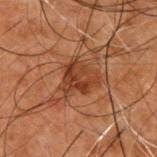The lesion was photographed on a routine skin check and not biopsied; there is no pathology result.
On the upper back.
A male subject, aged 48 to 52.
A roughly 15 mm field-of-view crop from a total-body skin photograph.
The tile uses cross-polarized illumination.
The lesion's longest dimension is about 4 mm.
The lesion-visualizer software estimated an average lesion color of about L≈31 a*≈21 b*≈28 (CIELAB), about 7 CIELAB-L* units darker than the surrounding skin, and a lesion-to-skin contrast of about 7.5 (normalized; higher = more distinct). And it measured a border-irregularity index near 4/10.